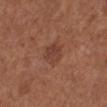Q: Who is the patient?
A: female, aged approximately 55
Q: What kind of image is this?
A: 15 mm crop, total-body photography
Q: What is the lesion's diameter?
A: ≈3 mm
Q: What is the anatomic site?
A: the left lower leg